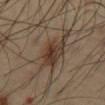Assessment: The lesion was photographed on a routine skin check and not biopsied; there is no pathology result. Context: Cropped from a total-body skin-imaging series; the visible field is about 15 mm. On the front of the torso. The patient is a male in their 40s.